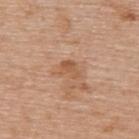follow-up=total-body-photography surveillance lesion; no biopsy | lighting=white-light | patient=female, roughly 65 years of age | body site=the upper back | image=~15 mm crop, total-body skin-cancer survey.